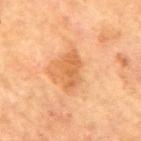The lesion was tiled from a total-body skin photograph and was not biopsied. The tile uses cross-polarized illumination. An algorithmic analysis of the crop reported a classifier nevus-likeness of about 0/100 and a detector confidence of about 100 out of 100 that the crop contains a lesion. The patient is a female about 70 years old. A region of skin cropped from a whole-body photographic capture, roughly 15 mm wide. Longest diameter approximately 4.5 mm. Located on the right upper arm.